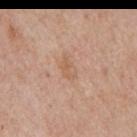Captured during whole-body skin photography for melanoma surveillance; the lesion was not biopsied.
The patient is a male approximately 55 years of age.
This image is a 15 mm lesion crop taken from a total-body photograph.
The lesion is located on the mid back.